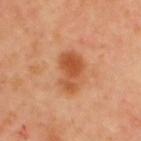Captured during whole-body skin photography for melanoma surveillance; the lesion was not biopsied. The patient is a male in their mid-60s. An algorithmic analysis of the crop reported a within-lesion color-variation index near 4.5/10. Located on the back. The tile uses cross-polarized illumination. Cropped from a total-body skin-imaging series; the visible field is about 15 mm.